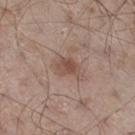Notes:
- notes · total-body-photography surveillance lesion; no biopsy
- anatomic site · the right thigh
- imaging modality · 15 mm crop, total-body photography
- subject · male, aged 53–57
- automated lesion analysis · an area of roughly 4.5 mm² and two-axis asymmetry of about 0.25; a normalized border contrast of about 7; a nevus-likeness score of about 50/100 and lesion-presence confidence of about 100/100